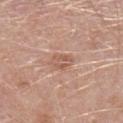Captured during whole-body skin photography for melanoma surveillance; the lesion was not biopsied. The patient is a male in their 50s. Imaged with white-light lighting. A 15 mm close-up extracted from a 3D total-body photography capture. About 3 mm across. From the left lower leg.